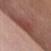Impression: Part of a total-body skin-imaging series; this lesion was reviewed on a skin check and was not flagged for biopsy. Background: Automated tile analysis of the lesion measured a footprint of about 14 mm², a shape eccentricity near 0.8, and a shape-asymmetry score of about 0.3 (0 = symmetric). Imaged with white-light lighting. A close-up tile cropped from a whole-body skin photograph, about 15 mm across. A female patient aged approximately 65. Approximately 6 mm at its widest. Located on the front of the torso.